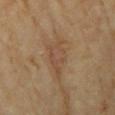Impression:
Part of a total-body skin-imaging series; this lesion was reviewed on a skin check and was not flagged for biopsy.
Acquisition and patient details:
A close-up tile cropped from a whole-body skin photograph, about 15 mm across. Longest diameter approximately 4.5 mm. The tile uses cross-polarized illumination. Located on the left upper arm. A female patient about 60 years old.